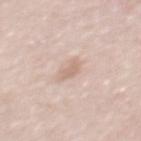The lesion was photographed on a routine skin check and not biopsied; there is no pathology result.
The lesion is located on the mid back.
Imaged with white-light lighting.
A male subject, aged around 50.
Cropped from a total-body skin-imaging series; the visible field is about 15 mm.
Approximately 3 mm at its widest.